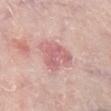site: right lower leg
image:
  source: total-body photography crop
  field_of_view_mm: 15
patient:
  sex: female
  age_approx: 65
lighting: white-light